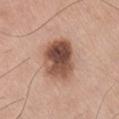biopsy_status: not biopsied; imaged during a skin examination